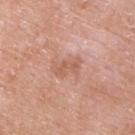No biopsy was performed on this lesion — it was imaged during a full skin examination and was not determined to be concerning. The subject is a male roughly 75 years of age. The total-body-photography lesion software estimated an eccentricity of roughly 0.85 and a symmetry-axis asymmetry near 0.35. And it measured a lesion color around L≈59 a*≈23 b*≈30 in CIELAB and a normalized lesion–skin contrast near 5.5. A lesion tile, about 15 mm wide, cut from a 3D total-body photograph. The lesion is on the upper back.